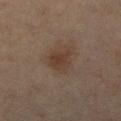Findings:
* location: the right lower leg
* size: about 3.5 mm
* patient: female, approximately 60 years of age
* lighting: cross-polarized illumination
* acquisition: total-body-photography crop, ~15 mm field of view